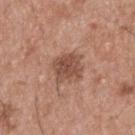biopsy status: imaged on a skin check; not biopsied
diameter: about 3.5 mm
image source: 15 mm crop, total-body photography
TBP lesion metrics: an area of roughly 9.5 mm², an outline eccentricity of about 0.15 (0 = round, 1 = elongated), and a symmetry-axis asymmetry near 0.15; a mean CIELAB color near L≈50 a*≈21 b*≈28, a lesion–skin lightness drop of about 11, and a normalized border contrast of about 7.5; a border-irregularity index near 2/10, a within-lesion color-variation index near 3/10, and peripheral color asymmetry of about 1
illumination: white-light illumination
site: the back
patient: male, aged around 55